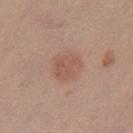Background: Approximately 4 mm at its widest. A lesion tile, about 15 mm wide, cut from a 3D total-body photograph. The tile uses white-light illumination. Located on the leg. The subject is a female aged 38 to 42.